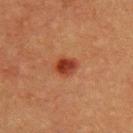Findings:
* biopsy status: no biopsy performed (imaged during a skin exam)
* acquisition: ~15 mm tile from a whole-body skin photo
* patient: male, in their 40s
* site: the upper back